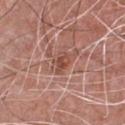Recorded during total-body skin imaging; not selected for excision or biopsy. The lesion is located on the chest. The tile uses white-light illumination. A male subject approximately 65 years of age. A 15 mm close-up extracted from a 3D total-body photography capture. Approximately 3 mm at its widest.